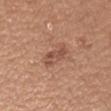Q: Is there a histopathology result?
A: total-body-photography surveillance lesion; no biopsy
Q: How was this image acquired?
A: ~15 mm crop, total-body skin-cancer survey
Q: What is the anatomic site?
A: the right forearm
Q: What are the patient's age and sex?
A: female, aged around 45
Q: Automated lesion metrics?
A: a detector confidence of about 100 out of 100 that the crop contains a lesion
Q: Lesion size?
A: ~4 mm (longest diameter)
Q: What lighting was used for the tile?
A: white-light